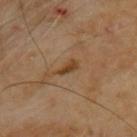Impression:
Part of a total-body skin-imaging series; this lesion was reviewed on a skin check and was not flagged for biopsy.
Clinical summary:
The lesion is on the back. Cropped from a total-body skin-imaging series; the visible field is about 15 mm. The recorded lesion diameter is about 3 mm. This is a cross-polarized tile. The patient is a male aged approximately 70.